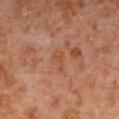notes = no biopsy performed (imaged during a skin exam)
automated lesion analysis = a lesion area of about 3 mm²; internal color variation of about 1 on a 0–10 scale; a lesion-detection confidence of about 100/100
location = the left lower leg
lesion diameter = about 2.5 mm
image source = 15 mm crop, total-body photography
lighting = cross-polarized
patient = male, in their 30s